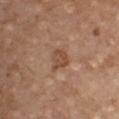patient: male, about 70 years old
image-analysis metrics: a lesion color around L≈48 a*≈21 b*≈30 in CIELAB and roughly 9 lightness units darker than nearby skin
lighting: white-light illumination
image: 15 mm crop, total-body photography
anatomic site: the chest
size: ≈2.5 mm